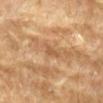Q: Was a biopsy performed?
A: total-body-photography surveillance lesion; no biopsy
Q: What is the anatomic site?
A: the left forearm
Q: What is the imaging modality?
A: 15 mm crop, total-body photography
Q: Who is the patient?
A: male, approximately 85 years of age or older
Q: How large is the lesion?
A: ≈2.5 mm
Q: What lighting was used for the tile?
A: cross-polarized illumination